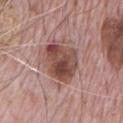TBP lesion metrics: two-axis asymmetry of about 0.2; a mean CIELAB color near L≈47 a*≈22 b*≈24 and a lesion-to-skin contrast of about 9.5 (normalized; higher = more distinct); a nevus-likeness score of about 80/100 and a detector confidence of about 100 out of 100 that the crop contains a lesion
body site: the chest
illumination: white-light illumination
patient: male, about 70 years old
lesion size: about 5 mm
imaging modality: ~15 mm crop, total-body skin-cancer survey